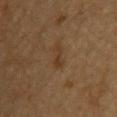follow-up: catalogued during a skin exam; not biopsied | location: the back | imaging modality: 15 mm crop, total-body photography | patient: male, about 85 years old or older.